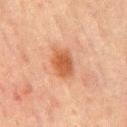Imaged during a routine full-body skin examination; the lesion was not biopsied and no histopathology is available. Located on the mid back. Imaged with cross-polarized lighting. The subject is a male in their mid-60s. A roughly 15 mm field-of-view crop from a total-body skin photograph.